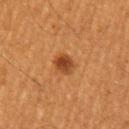Imaged during a routine full-body skin examination; the lesion was not biopsied and no histopathology is available. A 15 mm crop from a total-body photograph taken for skin-cancer surveillance. On the arm. A male subject aged 58 to 62.